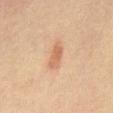{
  "biopsy_status": "not biopsied; imaged during a skin examination",
  "image": {
    "source": "total-body photography crop",
    "field_of_view_mm": 15
  },
  "lesion_size": {
    "long_diameter_mm_approx": 3.5
  },
  "site": "front of the torso",
  "patient": {
    "sex": "female",
    "age_approx": 65
  },
  "automated_metrics": {
    "shape_asymmetry": 0.25
  }
}